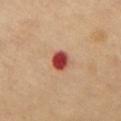notes = total-body-photography surveillance lesion; no biopsy | patient = female, aged 53–57 | imaging modality = ~15 mm tile from a whole-body skin photo | location = the front of the torso.